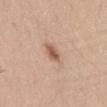| key | value |
|---|---|
| follow-up | no biopsy performed (imaged during a skin exam) |
| acquisition | 15 mm crop, total-body photography |
| site | the abdomen |
| patient | male, aged 43 to 47 |
| diameter | about 2.5 mm |
| illumination | white-light illumination |
| TBP lesion metrics | a footprint of about 3.5 mm² and a symmetry-axis asymmetry near 0.25; an average lesion color of about L≈57 a*≈21 b*≈31 (CIELAB), roughly 12 lightness units darker than nearby skin, and a lesion-to-skin contrast of about 8 (normalized; higher = more distinct); a color-variation rating of about 2/10 and a peripheral color-asymmetry measure near 0.5; a classifier nevus-likeness of about 85/100 and a lesion-detection confidence of about 100/100 |